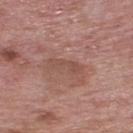The lesion was tiled from a total-body skin photograph and was not biopsied. A male patient aged approximately 65. Longest diameter approximately 3.5 mm. Located on the back. Automated tile analysis of the lesion measured border irregularity of about 5 on a 0–10 scale, a within-lesion color-variation index near 2.5/10, and peripheral color asymmetry of about 0.5. A 15 mm close-up extracted from a 3D total-body photography capture. The tile uses white-light illumination.